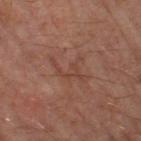Q: Is there a histopathology result?
A: no biopsy performed (imaged during a skin exam)
Q: Patient demographics?
A: male, aged around 65
Q: What is the anatomic site?
A: the leg
Q: How was this image acquired?
A: ~15 mm tile from a whole-body skin photo